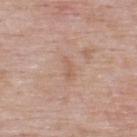Notes:
• biopsy status — catalogued during a skin exam; not biopsied
• body site — the upper back
• patient — male, in their mid-50s
• automated metrics — a symmetry-axis asymmetry near 0.35; a border-irregularity rating of about 3.5/10, internal color variation of about 0 on a 0–10 scale, and radial color variation of about 0
• diameter — about 2.5 mm
• acquisition — ~15 mm crop, total-body skin-cancer survey
• tile lighting — white-light illumination